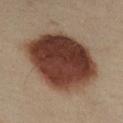notes = imaged on a skin check; not biopsied | anatomic site = the arm | patient = female, roughly 40 years of age | size = about 8.5 mm | image = ~15 mm tile from a whole-body skin photo | illumination = cross-polarized.